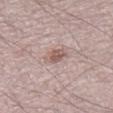follow-up: catalogued during a skin exam; not biopsied
illumination: white-light
image: total-body-photography crop, ~15 mm field of view
lesion diameter: about 3 mm
subject: male, aged approximately 50
site: the right thigh